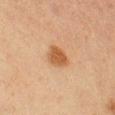This lesion was catalogued during total-body skin photography and was not selected for biopsy. A male patient roughly 35 years of age. Located on the chest. The total-body-photography lesion software estimated a lesion color around L≈51 a*≈21 b*≈36 in CIELAB and a normalized lesion–skin contrast near 8.5. And it measured an automated nevus-likeness rating near 100 out of 100. Longest diameter approximately 3.5 mm. The tile uses cross-polarized illumination. Cropped from a total-body skin-imaging series; the visible field is about 15 mm.